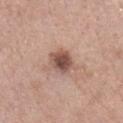biopsy_status: not biopsied; imaged during a skin examination
site: left forearm
lighting: white-light
lesion_size:
  long_diameter_mm_approx: 3.5
patient:
  sex: female
  age_approx: 55
image:
  source: total-body photography crop
  field_of_view_mm: 15